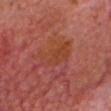Assessment:
This lesion was catalogued during total-body skin photography and was not selected for biopsy.